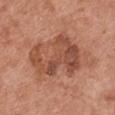• follow-up: no biopsy performed (imaged during a skin exam)
• patient: female, approximately 65 years of age
• body site: the chest
• acquisition: total-body-photography crop, ~15 mm field of view